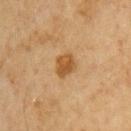Q: Is there a histopathology result?
A: no biopsy performed (imaged during a skin exam)
Q: How was this image acquired?
A: total-body-photography crop, ~15 mm field of view
Q: Where on the body is the lesion?
A: the left upper arm
Q: Who is the patient?
A: female, aged around 55
Q: What lighting was used for the tile?
A: cross-polarized
Q: What is the lesion's diameter?
A: ≈2.5 mm